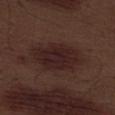workup: catalogued during a skin exam; not biopsied
lesion size: about 7 mm
automated metrics: a lesion area of about 21 mm² and a shape-asymmetry score of about 0.15 (0 = symmetric); border irregularity of about 2.5 on a 0–10 scale and a within-lesion color-variation index near 3/10
tile lighting: white-light
patient: male, approximately 70 years of age
body site: the left thigh
imaging modality: total-body-photography crop, ~15 mm field of view